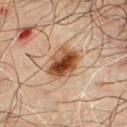follow-up = total-body-photography surveillance lesion; no biopsy
body site = the chest
image = ~15 mm crop, total-body skin-cancer survey
tile lighting = cross-polarized
lesion diameter = ≈4.5 mm
patient = male, in their 70s